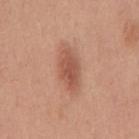Clinical impression:
Imaged during a routine full-body skin examination; the lesion was not biopsied and no histopathology is available.
Image and clinical context:
Automated image analysis of the tile measured a footprint of about 11 mm², a shape eccentricity near 0.85, and two-axis asymmetry of about 0.1. The software also gave a lesion–skin lightness drop of about 10 and a normalized border contrast of about 7. It also reported a border-irregularity rating of about 2/10, a color-variation rating of about 3/10, and a peripheral color-asymmetry measure near 1. This is a white-light tile. On the mid back. Cropped from a whole-body photographic skin survey; the tile spans about 15 mm. The patient is a male roughly 50 years of age.